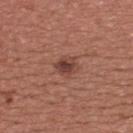No biopsy was performed on this lesion — it was imaged during a full skin examination and was not determined to be concerning.
A male subject aged 38–42.
The lesion's longest dimension is about 3 mm.
Imaged with white-light lighting.
A close-up tile cropped from a whole-body skin photograph, about 15 mm across.
On the upper back.